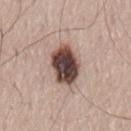{
  "biopsy_status": "not biopsied; imaged during a skin examination",
  "patient": {
    "sex": "male",
    "age_approx": 75
  },
  "site": "lower back",
  "lesion_size": {
    "long_diameter_mm_approx": 5.0
  },
  "image": {
    "source": "total-body photography crop",
    "field_of_view_mm": 15
  },
  "automated_metrics": {
    "cielab_L": 44,
    "cielab_a": 17,
    "cielab_b": 22,
    "vs_skin_darker_L": 24.0,
    "vs_skin_contrast_norm": 16.0,
    "nevus_likeness_0_100": 80,
    "lesion_detection_confidence_0_100": 100
  },
  "lighting": "white-light"
}